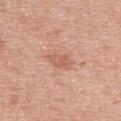Q: Was a biopsy performed?
A: catalogued during a skin exam; not biopsied
Q: What kind of image is this?
A: ~15 mm tile from a whole-body skin photo
Q: Lesion location?
A: the back
Q: Lesion size?
A: ≈3 mm
Q: Illumination type?
A: white-light illumination
Q: What did automated image analysis measure?
A: an area of roughly 4.5 mm², a shape eccentricity near 0.8, and a shape-asymmetry score of about 0.3 (0 = symmetric); a border-irregularity index near 3/10 and a color-variation rating of about 2.5/10
Q: Patient demographics?
A: male, aged 63–67